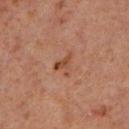workup: no biopsy performed (imaged during a skin exam)
acquisition: ~15 mm tile from a whole-body skin photo
TBP lesion metrics: a footprint of about 4 mm², a shape eccentricity near 0.7, and a shape-asymmetry score of about 0.65 (0 = symmetric); a border-irregularity index near 6.5/10, a within-lesion color-variation index near 2/10, and a peripheral color-asymmetry measure near 0.5; a nevus-likeness score of about 0/100
location: the left lower leg
patient: male, aged approximately 60